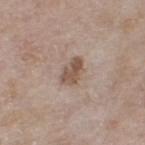{"biopsy_status": "not biopsied; imaged during a skin examination", "site": "left thigh", "lighting": "white-light", "patient": {"sex": "female", "age_approx": 75}, "image": {"source": "total-body photography crop", "field_of_view_mm": 15}, "lesion_size": {"long_diameter_mm_approx": 3.5}}